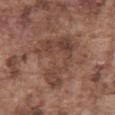workup: no biopsy performed (imaged during a skin exam) | subject: male, aged around 75 | illumination: white-light | site: the abdomen | lesion size: ~7 mm (longest diameter) | automated lesion analysis: a lesion area of about 18 mm² and a shape-asymmetry score of about 0.7 (0 = symmetric); an average lesion color of about L≈42 a*≈19 b*≈25 (CIELAB), about 8 CIELAB-L* units darker than the surrounding skin, and a normalized lesion–skin contrast near 7; radial color variation of about 1.5 | image: 15 mm crop, total-body photography.